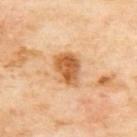Q: Was a biopsy performed?
A: imaged on a skin check; not biopsied
Q: Who is the patient?
A: female, approximately 60 years of age
Q: Lesion size?
A: ≈4 mm
Q: How was this image acquired?
A: ~15 mm tile from a whole-body skin photo
Q: Automated lesion metrics?
A: an outline eccentricity of about 0.65 (0 = round, 1 = elongated) and a symmetry-axis asymmetry near 0.2; a border-irregularity rating of about 2/10, internal color variation of about 5 on a 0–10 scale, and a peripheral color-asymmetry measure near 1.5; an automated nevus-likeness rating near 95 out of 100
Q: What is the anatomic site?
A: the upper back